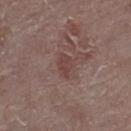{"site": "leg", "image": {"source": "total-body photography crop", "field_of_view_mm": 15}, "automated_metrics": {"border_irregularity_0_10": 3.0, "color_variation_0_10": 1.0, "peripheral_color_asymmetry": 0.5, "lesion_detection_confidence_0_100": 100}, "lesion_size": {"long_diameter_mm_approx": 3.0}, "lighting": "white-light", "patient": {"sex": "female", "age_approx": "85+"}}